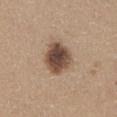Recorded during total-body skin imaging; not selected for excision or biopsy. A roughly 15 mm field-of-view crop from a total-body skin photograph. Located on the abdomen. The subject is a male aged 63 to 67.